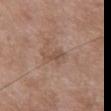Assessment:
Captured during whole-body skin photography for melanoma surveillance; the lesion was not biopsied.
Clinical summary:
The patient is a female aged 68 to 72. Automated tile analysis of the lesion measured a border-irregularity index near 4/10, a within-lesion color-variation index near 2/10, and peripheral color asymmetry of about 0.5. This image is a 15 mm lesion crop taken from a total-body photograph. The lesion is located on the chest. Imaged with white-light lighting.